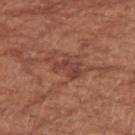{"biopsy_status": "not biopsied; imaged during a skin examination"}